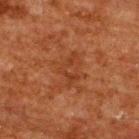Notes:
– workup · imaged on a skin check; not biopsied
– diameter · about 3.5 mm
– anatomic site · the back
– subject · male, aged around 60
– image · ~15 mm crop, total-body skin-cancer survey
– tile lighting · cross-polarized illumination